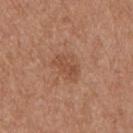No biopsy was performed on this lesion — it was imaged during a full skin examination and was not determined to be concerning.
The subject is a male in their mid-50s.
An algorithmic analysis of the crop reported border irregularity of about 2.5 on a 0–10 scale and a peripheral color-asymmetry measure near 1.
The lesion is on the left upper arm.
Imaged with white-light lighting.
Longest diameter approximately 3.5 mm.
Cropped from a total-body skin-imaging series; the visible field is about 15 mm.